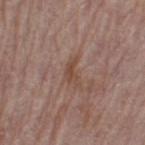follow-up = imaged on a skin check; not biopsied
illumination = white-light illumination
image source = total-body-photography crop, ~15 mm field of view
body site = the leg
size = about 3.5 mm
patient = female, aged 63 to 67